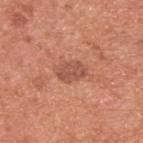No biopsy was performed on this lesion — it was imaged during a full skin examination and was not determined to be concerning. The subject is a male about 55 years old. The lesion's longest dimension is about 3 mm. A 15 mm close-up tile from a total-body photography series done for melanoma screening. The lesion is located on the back. Imaged with white-light lighting. An algorithmic analysis of the crop reported a footprint of about 6 mm², a shape eccentricity near 0.75, and a shape-asymmetry score of about 0.2 (0 = symmetric). The software also gave a border-irregularity rating of about 2/10 and a color-variation rating of about 3/10. The analysis additionally found a classifier nevus-likeness of about 10/100 and a lesion-detection confidence of about 100/100.